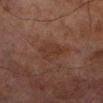Imaged with cross-polarized lighting. Located on the left lower leg. A 15 mm crop from a total-body photograph taken for skin-cancer surveillance. The patient is a male aged approximately 70.